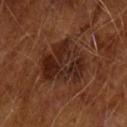workup: total-body-photography surveillance lesion; no biopsy
subject: male, aged 63–67
acquisition: 15 mm crop, total-body photography